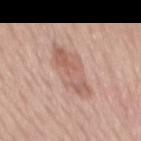This lesion was catalogued during total-body skin photography and was not selected for biopsy. Imaged with white-light lighting. A female patient in their mid- to late 50s. A 15 mm close-up tile from a total-body photography series done for melanoma screening. From the mid back. The total-body-photography lesion software estimated a footprint of about 14 mm², an eccentricity of roughly 0.9, and a shape-asymmetry score of about 0.25 (0 = symmetric). And it measured an average lesion color of about L≈60 a*≈21 b*≈26 (CIELAB) and a normalized lesion–skin contrast near 6. Longest diameter approximately 6.5 mm.